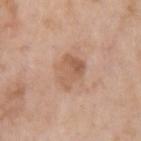notes = catalogued during a skin exam; not biopsied | subject = male, aged approximately 60 | site = the left upper arm | image source = total-body-photography crop, ~15 mm field of view.